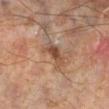workup: total-body-photography surveillance lesion; no biopsy | acquisition: total-body-photography crop, ~15 mm field of view | location: the left lower leg | subject: male, in their mid- to late 60s | lighting: cross-polarized illumination | size: ≈4 mm.